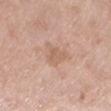notes: no biopsy performed (imaged during a skin exam)
anatomic site: the left lower leg
automated metrics: an average lesion color of about L≈61 a*≈19 b*≈29 (CIELAB), about 7 CIELAB-L* units darker than the surrounding skin, and a lesion-to-skin contrast of about 5 (normalized; higher = more distinct); an automated nevus-likeness rating near 0 out of 100 and a lesion-detection confidence of about 100/100
patient: female, approximately 70 years of age
imaging modality: ~15 mm tile from a whole-body skin photo
lesion diameter: ≈3 mm
illumination: white-light illumination